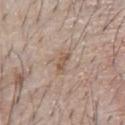notes: catalogued during a skin exam; not biopsied
image source: 15 mm crop, total-body photography
patient: male, about 65 years old
automated lesion analysis: a lesion color around L≈55 a*≈16 b*≈27 in CIELAB, roughly 9 lightness units darker than nearby skin, and a normalized lesion–skin contrast near 6.5
lesion size: ≈3 mm
site: the chest
lighting: white-light illumination